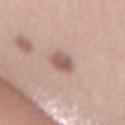Background: On the back. The subject is a female approximately 65 years of age. A 15 mm close-up tile from a total-body photography series done for melanoma screening.